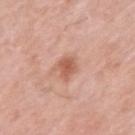biopsy status: no biopsy performed (imaged during a skin exam)
body site: the left upper arm
acquisition: total-body-photography crop, ~15 mm field of view
tile lighting: white-light illumination
size: about 3 mm
subject: male, approximately 80 years of age